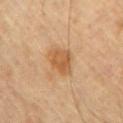Notes:
* notes: catalogued during a skin exam; not biopsied
* patient: male, in their mid- to late 60s
* TBP lesion metrics: a lesion color around L≈54 a*≈20 b*≈38 in CIELAB, a lesion–skin lightness drop of about 9, and a normalized border contrast of about 7.5; a border-irregularity index near 2.5/10, a color-variation rating of about 2.5/10, and radial color variation of about 1
* lighting: cross-polarized
* image: ~15 mm tile from a whole-body skin photo
* anatomic site: the chest
* lesion diameter: about 3.5 mm